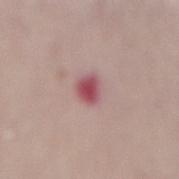The patient is a female aged 53–57. A roughly 15 mm field-of-view crop from a total-body skin photograph. Captured under white-light illumination. From the mid back.